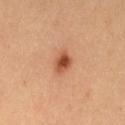Imaged during a routine full-body skin examination; the lesion was not biopsied and no histopathology is available.
The tile uses cross-polarized illumination.
The subject is a female roughly 35 years of age.
Measured at roughly 3 mm in maximum diameter.
From the left thigh.
A region of skin cropped from a whole-body photographic capture, roughly 15 mm wide.